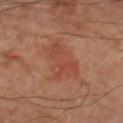Part of a total-body skin-imaging series; this lesion was reviewed on a skin check and was not flagged for biopsy.
The subject is a male in their 70s.
A roughly 15 mm field-of-view crop from a total-body skin photograph.
The lesion is on the left forearm.
Longest diameter approximately 5.5 mm.
Captured under cross-polarized illumination.
Automated image analysis of the tile measured a classifier nevus-likeness of about 5/100 and lesion-presence confidence of about 100/100.